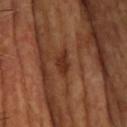Context:
A 15 mm close-up tile from a total-body photography series done for melanoma screening. Located on the head or neck. The lesion's longest dimension is about 3 mm.